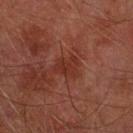| field | value |
|---|---|
| automated metrics | a lesion area of about 4.5 mm², a shape eccentricity near 0.7, and a symmetry-axis asymmetry near 0.35; an average lesion color of about L≈26 a*≈23 b*≈24 (CIELAB) and a lesion-to-skin contrast of about 6 (normalized; higher = more distinct); border irregularity of about 3.5 on a 0–10 scale and a peripheral color-asymmetry measure near 0.5 |
| lighting | cross-polarized |
| anatomic site | the arm |
| lesion size | about 2.5 mm |
| acquisition | ~15 mm tile from a whole-body skin photo |
| subject | male, in their mid- to late 70s |